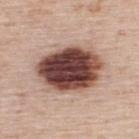Q: Was this lesion biopsied?
A: total-body-photography surveillance lesion; no biopsy
Q: How was this image acquired?
A: ~15 mm tile from a whole-body skin photo
Q: What lighting was used for the tile?
A: white-light illumination
Q: Patient demographics?
A: male, aged 73 to 77
Q: What is the anatomic site?
A: the back